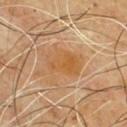The lesion was tiled from a total-body skin photograph and was not biopsied.